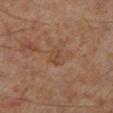workup = catalogued during a skin exam; not biopsied
subject = male, aged 58 to 62
size = ~2.5 mm (longest diameter)
image = 15 mm crop, total-body photography
body site = the leg